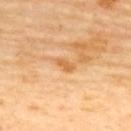  biopsy_status: not biopsied; imaged during a skin examination
  site: upper back
  lighting: cross-polarized
  automated_metrics:
    area_mm2_approx: 3.0
    eccentricity: 0.85
    cielab_L: 66
    cielab_a: 24
    cielab_b: 45
    vs_skin_darker_L: 10.0
    vs_skin_contrast_norm: 6.5
    border_irregularity_0_10: 3.0
    color_variation_0_10: 0.5
  image:
    source: total-body photography crop
    field_of_view_mm: 15
  lesion_size:
    long_diameter_mm_approx: 2.5
  patient:
    sex: female
    age_approx: 60